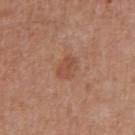Impression:
Part of a total-body skin-imaging series; this lesion was reviewed on a skin check and was not flagged for biopsy.
Acquisition and patient details:
The lesion is on the chest. A 15 mm close-up extracted from a 3D total-body photography capture. A male subject roughly 65 years of age.